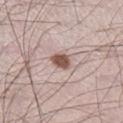{
  "biopsy_status": "not biopsied; imaged during a skin examination",
  "lesion_size": {
    "long_diameter_mm_approx": 2.5
  },
  "patient": {
    "sex": "male",
    "age_approx": 35
  },
  "site": "right lower leg",
  "image": {
    "source": "total-body photography crop",
    "field_of_view_mm": 15
  },
  "lighting": "white-light",
  "automated_metrics": {
    "vs_skin_contrast_norm": 11.0
  }
}